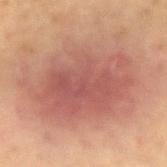Findings:
• notes: catalogued during a skin exam; not biopsied
• body site: the right forearm
• automated lesion analysis: a classifier nevus-likeness of about 75/100 and a detector confidence of about 100 out of 100 that the crop contains a lesion
• diameter: about 11.5 mm
• image source: ~15 mm tile from a whole-body skin photo
• patient: male, aged approximately 65
• illumination: cross-polarized